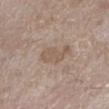workup: total-body-photography surveillance lesion; no biopsy | body site: the left lower leg | subject: female, aged 53–57 | imaging modality: ~15 mm tile from a whole-body skin photo | size: about 4 mm | automated lesion analysis: an area of roughly 7.5 mm², a shape eccentricity near 0.85, and two-axis asymmetry of about 0.35; roughly 7 lightness units darker than nearby skin and a lesion-to-skin contrast of about 5.5 (normalized; higher = more distinct); a classifier nevus-likeness of about 5/100 and a lesion-detection confidence of about 100/100.